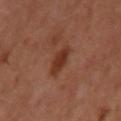<case>
  <biopsy_status>not biopsied; imaged during a skin examination</biopsy_status>
  <patient>
    <sex>male</sex>
    <age_approx>50</age_approx>
  </patient>
  <site>left upper arm</site>
  <lesion_size>
    <long_diameter_mm_approx>3.5</long_diameter_mm_approx>
  </lesion_size>
  <image>
    <source>total-body photography crop</source>
    <field_of_view_mm>15</field_of_view_mm>
  </image>
  <lighting>cross-polarized</lighting>
</case>